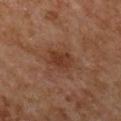Q: What is the lesion's diameter?
A: ≈3.5 mm
Q: What is the imaging modality?
A: ~15 mm crop, total-body skin-cancer survey
Q: What is the anatomic site?
A: the upper back
Q: What lighting was used for the tile?
A: cross-polarized illumination
Q: What are the patient's age and sex?
A: female, approximately 60 years of age